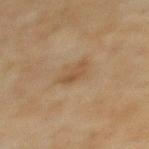A female patient, in their mid-50s.
A roughly 15 mm field-of-view crop from a total-body skin photograph.
Captured under cross-polarized illumination.
The total-body-photography lesion software estimated an area of roughly 3.5 mm², a shape eccentricity near 0.85, and a symmetry-axis asymmetry near 0.4. It also reported a color-variation rating of about 1.5/10.
Located on the back.
The lesion's longest dimension is about 3 mm.